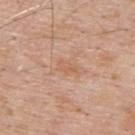The lesion was photographed on a routine skin check and not biopsied; there is no pathology result.
The total-body-photography lesion software estimated a lesion area of about 2.5 mm², a shape eccentricity near 0.85, and a symmetry-axis asymmetry near 0.35. And it measured a border-irregularity rating of about 3.5/10 and a peripheral color-asymmetry measure near 0. The software also gave lesion-presence confidence of about 100/100.
A lesion tile, about 15 mm wide, cut from a 3D total-body photograph.
The tile uses white-light illumination.
A male patient, aged around 60.
About 2.5 mm across.
The lesion is located on the upper back.